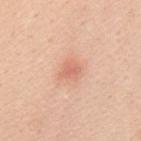Case summary:
– notes: imaged on a skin check; not biopsied
– acquisition: ~15 mm tile from a whole-body skin photo
– lesion diameter: ≈2.5 mm
– body site: the back
– patient: female, aged 43 to 47